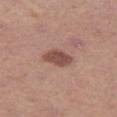workup = no biopsy performed (imaged during a skin exam) | anatomic site = the leg | subject = female, aged 38 to 42 | image = ~15 mm tile from a whole-body skin photo | size = ≈3.5 mm | automated metrics = border irregularity of about 2 on a 0–10 scale, a color-variation rating of about 2.5/10, and peripheral color asymmetry of about 1; an automated nevus-likeness rating near 90 out of 100 and lesion-presence confidence of about 100/100 | illumination = white-light illumination.